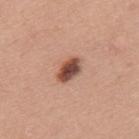biopsy status = imaged on a skin check; not biopsied | image = ~15 mm tile from a whole-body skin photo | patient = male, aged 38 to 42 | location = the upper back.